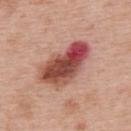Findings:
* tile lighting — white-light
* site — the upper back
* imaging modality — ~15 mm tile from a whole-body skin photo
* subject — male, in their mid-60s
* size — ~7 mm (longest diameter)